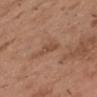Cropped from a total-body skin-imaging series; the visible field is about 15 mm. Captured under white-light illumination. The patient is a male about 55 years old. Longest diameter approximately 3 mm. The lesion is located on the head or neck.